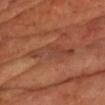Q: Is there a histopathology result?
A: total-body-photography surveillance lesion; no biopsy
Q: What is the lesion's diameter?
A: ≈5.5 mm
Q: What is the imaging modality?
A: total-body-photography crop, ~15 mm field of view
Q: Where on the body is the lesion?
A: the chest
Q: Patient demographics?
A: male, aged around 65
Q: What did automated image analysis measure?
A: an area of roughly 9.5 mm² and a shape eccentricity near 0.9; an automated nevus-likeness rating near 0 out of 100